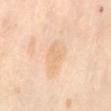The lesion was tiled from a total-body skin photograph and was not biopsied.
The lesion is located on the mid back.
A female patient in their mid- to late 50s.
A 15 mm close-up extracted from a 3D total-body photography capture.